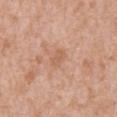  biopsy_status: not biopsied; imaged during a skin examination
  patient:
    sex: male
    age_approx: 60
  lesion_size:
    long_diameter_mm_approx: 2.5
  image:
    source: total-body photography crop
    field_of_view_mm: 15
  site: mid back
  lighting: white-light
  automated_metrics:
    cielab_L: 60
    cielab_a: 21
    cielab_b: 33
    vs_skin_contrast_norm: 5.0